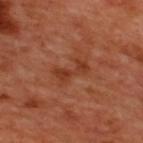Assessment:
The lesion was tiled from a total-body skin photograph and was not biopsied.
Image and clinical context:
On the upper back. Captured under cross-polarized illumination. The lesion's longest dimension is about 4 mm. A 15 mm close-up tile from a total-body photography series done for melanoma screening. A male patient, aged around 50.